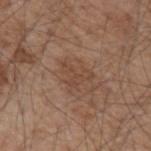Clinical impression:
Recorded during total-body skin imaging; not selected for excision or biopsy.
Background:
The tile uses white-light illumination. Approximately 3.5 mm at its widest. A lesion tile, about 15 mm wide, cut from a 3D total-body photograph. A male patient, aged approximately 55. On the arm.